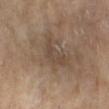lesion size = ≈4 mm | acquisition = total-body-photography crop, ~15 mm field of view | patient = female, aged 73 to 77 | TBP lesion metrics = an area of roughly 5.5 mm², an eccentricity of roughly 0.85, and two-axis asymmetry of about 0.45; an average lesion color of about L≈44 a*≈14 b*≈26 (CIELAB) and about 6 CIELAB-L* units darker than the surrounding skin; a classifier nevus-likeness of about 0/100 and lesion-presence confidence of about 100/100 | tile lighting = cross-polarized | body site = the left lower leg.